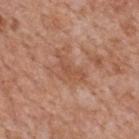| key | value |
|---|---|
| notes | no biopsy performed (imaged during a skin exam) |
| anatomic site | the mid back |
| lesion size | about 4.5 mm |
| illumination | white-light |
| TBP lesion metrics | a footprint of about 6.5 mm², an eccentricity of roughly 0.85, and a shape-asymmetry score of about 0.55 (0 = symmetric); a lesion color around L≈52 a*≈23 b*≈32 in CIELAB, a lesion–skin lightness drop of about 7, and a normalized lesion–skin contrast near 5; a border-irregularity rating of about 6/10, a color-variation rating of about 1.5/10, and peripheral color asymmetry of about 0.5 |
| patient | male, aged approximately 65 |
| imaging modality | ~15 mm crop, total-body skin-cancer survey |